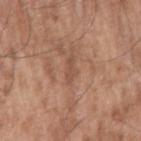Clinical impression: No biopsy was performed on this lesion — it was imaged during a full skin examination and was not determined to be concerning. Clinical summary: The lesion is on the arm. Cropped from a whole-body photographic skin survey; the tile spans about 15 mm. A male subject in their mid- to late 50s. Automated image analysis of the tile measured an area of roughly 2 mm² and a shape-asymmetry score of about 0.35 (0 = symmetric). The analysis additionally found a color-variation rating of about 0/10 and peripheral color asymmetry of about 0. The recorded lesion diameter is about 2.5 mm. This is a white-light tile.